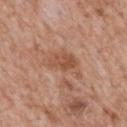biopsy status: no biopsy performed (imaged during a skin exam)
patient: male, aged 63–67
image-analysis metrics: a lesion area of about 10 mm², an eccentricity of roughly 0.85, and a symmetry-axis asymmetry near 0.4; an automated nevus-likeness rating near 5 out of 100 and a lesion-detection confidence of about 100/100
lighting: white-light illumination
body site: the mid back
acquisition: total-body-photography crop, ~15 mm field of view